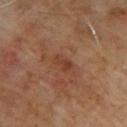| key | value |
|---|---|
| biopsy status | total-body-photography surveillance lesion; no biopsy |
| subject | male, aged 63–67 |
| lesion diameter | ~2.5 mm (longest diameter) |
| image-analysis metrics | a footprint of about 3 mm², an outline eccentricity of about 0.85 (0 = round, 1 = elongated), and two-axis asymmetry of about 0.25; a mean CIELAB color near L≈38 a*≈23 b*≈30, about 7 CIELAB-L* units darker than the surrounding skin, and a normalized border contrast of about 6; a lesion-detection confidence of about 100/100 |
| site | the back |
| imaging modality | 15 mm crop, total-body photography |
| lighting | cross-polarized illumination |